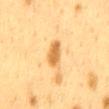No biopsy was performed on this lesion — it was imaged during a full skin examination and was not determined to be concerning. The recorded lesion diameter is about 4 mm. A region of skin cropped from a whole-body photographic capture, roughly 15 mm wide. A female subject roughly 40 years of age. This is a cross-polarized tile. On the mid back.